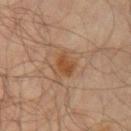This lesion was catalogued during total-body skin photography and was not selected for biopsy. This is a cross-polarized tile. Measured at roughly 2.5 mm in maximum diameter. The patient is a male aged around 65. An algorithmic analysis of the crop reported an average lesion color of about L≈44 a*≈21 b*≈34 (CIELAB), a lesion–skin lightness drop of about 8, and a lesion-to-skin contrast of about 8 (normalized; higher = more distinct). From the left upper arm. A roughly 15 mm field-of-view crop from a total-body skin photograph.